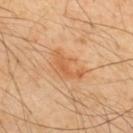Case summary:
* follow-up — no biopsy performed (imaged during a skin exam)